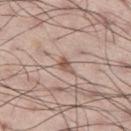follow-up = catalogued during a skin exam; not biopsied
subject = male, in their mid- to late 40s
image source = ~15 mm tile from a whole-body skin photo
site = the left thigh